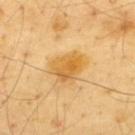| field | value |
|---|---|
| notes | total-body-photography surveillance lesion; no biopsy |